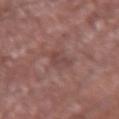The subject is a male aged around 65. The lesion-visualizer software estimated an area of roughly 3 mm² and a shape-asymmetry score of about 0.6 (0 = symmetric). Located on the right forearm. Imaged with white-light lighting. About 3.5 mm across. Cropped from a whole-body photographic skin survey; the tile spans about 15 mm.